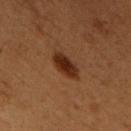Clinical impression:
Part of a total-body skin-imaging series; this lesion was reviewed on a skin check and was not flagged for biopsy.
Image and clinical context:
Cropped from a whole-body photographic skin survey; the tile spans about 15 mm. The lesion is on the chest. A male subject, approximately 50 years of age.